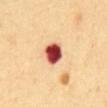<lesion>
<biopsy_status>not biopsied; imaged during a skin examination</biopsy_status>
<image>
  <source>total-body photography crop</source>
  <field_of_view_mm>15</field_of_view_mm>
</image>
<site>abdomen</site>
<patient>
  <sex>female</sex>
  <age_approx>50</age_approx>
</patient>
</lesion>